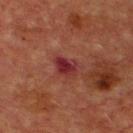<record>
<biopsy_status>not biopsied; imaged during a skin examination</biopsy_status>
<lesion_size>
  <long_diameter_mm_approx>3.0</long_diameter_mm_approx>
</lesion_size>
<image>
  <source>total-body photography crop</source>
  <field_of_view_mm>15</field_of_view_mm>
</image>
<patient>
  <sex>male</sex>
  <age_approx>65</age_approx>
</patient>
<site>chest</site>
<lighting>cross-polarized</lighting>
</record>